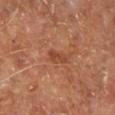{
  "biopsy_status": "not biopsied; imaged during a skin examination",
  "automated_metrics": {
    "eccentricity": 0.9,
    "shape_asymmetry": 0.3,
    "border_irregularity_0_10": 3.0,
    "peripheral_color_asymmetry": 0.5,
    "nevus_likeness_0_100": 0,
    "lesion_detection_confidence_0_100": 100
  },
  "image": {
    "source": "total-body photography crop",
    "field_of_view_mm": 15
  },
  "site": "right leg",
  "lighting": "cross-polarized",
  "lesion_size": {
    "long_diameter_mm_approx": 2.5
  },
  "patient": {
    "sex": "male",
    "age_approx": 60
  }
}